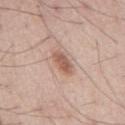Captured during whole-body skin photography for melanoma surveillance; the lesion was not biopsied. The lesion is located on the back. A close-up tile cropped from a whole-body skin photograph, about 15 mm across. A male patient about 55 years old. Approximately 3.5 mm at its widest. Automated tile analysis of the lesion measured a footprint of about 5 mm², an outline eccentricity of about 0.85 (0 = round, 1 = elongated), and two-axis asymmetry of about 0.2. The software also gave a border-irregularity rating of about 2/10, internal color variation of about 2.5 on a 0–10 scale, and a peripheral color-asymmetry measure near 1.